This image is a 15 mm lesion crop taken from a total-body photograph, on the leg, the subject is female — 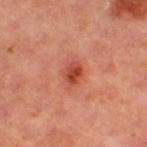The tile uses cross-polarized illumination.
Approximately 2.5 mm at its widest.
On biopsy, histopathology showed an indeterminate (borderline) lesion: atypical melanocytic neoplasm.